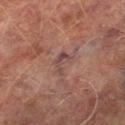Clinical impression: The lesion was tiled from a total-body skin photograph and was not biopsied. Acquisition and patient details: Approximately 2.5 mm at its widest. The total-body-photography lesion software estimated an area of roughly 3 mm², an eccentricity of roughly 0.8, and two-axis asymmetry of about 0.5. The analysis additionally found a border-irregularity rating of about 6/10, a within-lesion color-variation index near 2.5/10, and peripheral color asymmetry of about 1. The software also gave a classifier nevus-likeness of about 0/100 and a lesion-detection confidence of about 60/100. A male patient approximately 75 years of age. The lesion is located on the right lower leg. The tile uses cross-polarized illumination. Cropped from a total-body skin-imaging series; the visible field is about 15 mm.